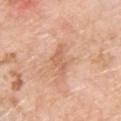Notes:
- workup — imaged on a skin check; not biopsied
- size — ≈4 mm
- automated lesion analysis — a mean CIELAB color near L≈63 a*≈23 b*≈33 and a normalized lesion–skin contrast near 5; internal color variation of about 1.5 on a 0–10 scale and peripheral color asymmetry of about 0.5
- subject — male, approximately 60 years of age
- tile lighting — white-light
- image — ~15 mm crop, total-body skin-cancer survey
- site — the upper back